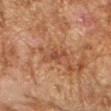follow-up: no biopsy performed (imaged during a skin exam); anatomic site: the right forearm; image: total-body-photography crop, ~15 mm field of view; patient: male, aged approximately 60.